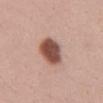Recorded during total-body skin imaging; not selected for excision or biopsy.
Captured under white-light illumination.
A 15 mm close-up extracted from a 3D total-body photography capture.
The lesion's longest dimension is about 5 mm.
A female patient approximately 35 years of age.
The lesion is located on the mid back.